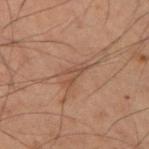Assessment: No biopsy was performed on this lesion — it was imaged during a full skin examination and was not determined to be concerning. Acquisition and patient details: A 15 mm close-up tile from a total-body photography series done for melanoma screening. The patient is a male aged approximately 60. The lesion is on the right upper arm. The tile uses cross-polarized illumination. Approximately 3.5 mm at its widest.